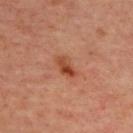workup: no biopsy performed (imaged during a skin exam)
imaging modality: total-body-photography crop, ~15 mm field of view
lighting: cross-polarized
patient: female, aged around 45
location: the upper back